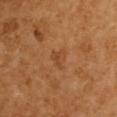Part of a total-body skin-imaging series; this lesion was reviewed on a skin check and was not flagged for biopsy.
The recorded lesion diameter is about 3 mm.
On the upper back.
The subject is a female aged 53 to 57.
A lesion tile, about 15 mm wide, cut from a 3D total-body photograph.
Automated image analysis of the tile measured an area of roughly 4 mm² and a symmetry-axis asymmetry near 0.45.